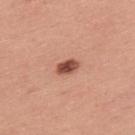The lesion was photographed on a routine skin check and not biopsied; there is no pathology result.
The lesion is on the back.
The patient is a male aged around 40.
A region of skin cropped from a whole-body photographic capture, roughly 15 mm wide.
Longest diameter approximately 3 mm.